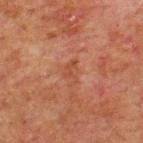  biopsy_status: not biopsied; imaged during a skin examination
  automated_metrics:
    area_mm2_approx: 3.0
    shape_asymmetry: 0.5
    cielab_L: 36
    cielab_a: 21
    cielab_b: 27
    vs_skin_contrast_norm: 5.0
    nevus_likeness_0_100: 0
    lesion_detection_confidence_0_100: 100
  patient:
    sex: male
    age_approx: 60
  lesion_size:
    long_diameter_mm_approx: 3.0
  site: back
  image:
    source: total-body photography crop
    field_of_view_mm: 15
  lighting: cross-polarized